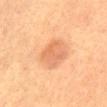Cropped from a total-body skin-imaging series; the visible field is about 15 mm.
Longest diameter approximately 3.5 mm.
The patient is a female aged 58–62.
On the abdomen.
The lesion-visualizer software estimated a footprint of about 11 mm², an outline eccentricity of about 0.4 (0 = round, 1 = elongated), and a symmetry-axis asymmetry near 0.15. It also reported a border-irregularity index near 1.5/10, a color-variation rating of about 3.5/10, and radial color variation of about 1.5. It also reported a nevus-likeness score of about 55/100 and a lesion-detection confidence of about 100/100.
The tile uses cross-polarized illumination.